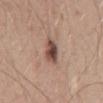biopsy_status: not biopsied; imaged during a skin examination
site: mid back
image:
  source: total-body photography crop
  field_of_view_mm: 15
patient:
  sex: male
  age_approx: 50